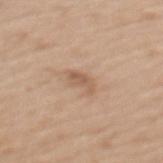{
  "biopsy_status": "not biopsied; imaged during a skin examination",
  "patient": {
    "sex": "female",
    "age_approx": 70
  },
  "image": {
    "source": "total-body photography crop",
    "field_of_view_mm": 15
  },
  "lesion_size": {
    "long_diameter_mm_approx": 3.5
  },
  "site": "mid back",
  "automated_metrics": {
    "area_mm2_approx": 4.0,
    "eccentricity": 0.9,
    "shape_asymmetry": 0.4,
    "cielab_L": 58,
    "cielab_a": 18,
    "cielab_b": 31,
    "vs_skin_contrast_norm": 6.0
  },
  "lighting": "white-light"
}